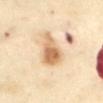A female patient, about 55 years old.
This is a cross-polarized tile.
The lesion is located on the abdomen.
Longest diameter approximately 5 mm.
A region of skin cropped from a whole-body photographic capture, roughly 15 mm wide.